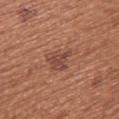Clinical impression:
The lesion was tiled from a total-body skin photograph and was not biopsied.
Background:
Cropped from a total-body skin-imaging series; the visible field is about 15 mm. The lesion's longest dimension is about 4 mm. A female subject roughly 50 years of age. The lesion-visualizer software estimated an average lesion color of about L≈47 a*≈22 b*≈28 (CIELAB), a lesion–skin lightness drop of about 8, and a lesion-to-skin contrast of about 6.5 (normalized; higher = more distinct). The software also gave a border-irregularity index near 4/10 and a within-lesion color-variation index near 2.5/10. Captured under white-light illumination. On the upper back.